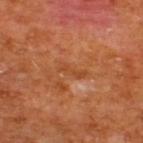<lesion>
  <biopsy_status>not biopsied; imaged during a skin examination</biopsy_status>
  <image>
    <source>total-body photography crop</source>
    <field_of_view_mm>15</field_of_view_mm>
  </image>
  <automated_metrics>
    <cielab_L>44</cielab_L>
    <cielab_a>27</cielab_a>
    <cielab_b>38</cielab_b>
    <vs_skin_darker_L>6.0</vs_skin_darker_L>
    <border_irregularity_0_10>4.0</border_irregularity_0_10>
    <peripheral_color_asymmetry>0.0</peripheral_color_asymmetry>
    <nevus_likeness_0_100>0</nevus_likeness_0_100>
  </automated_metrics>
  <lesion_size>
    <long_diameter_mm_approx>3.0</long_diameter_mm_approx>
  </lesion_size>
  <site>upper back</site>
  <patient>
    <sex>male</sex>
    <age_approx>65</age_approx>
  </patient>
  <lighting>cross-polarized</lighting>
</lesion>